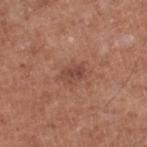Notes:
– diameter · ~2.5 mm (longest diameter)
– lighting · white-light
– automated lesion analysis · an area of roughly 3.5 mm², an outline eccentricity of about 0.8 (0 = round, 1 = elongated), and two-axis asymmetry of about 0.25
– imaging modality · ~15 mm crop, total-body skin-cancer survey
– subject · male, roughly 75 years of age
– anatomic site · the right lower leg